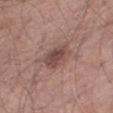Imaged during a routine full-body skin examination; the lesion was not biopsied and no histopathology is available. About 4.5 mm across. Automated image analysis of the tile measured a border-irregularity index near 2.5/10, a color-variation rating of about 3.5/10, and a peripheral color-asymmetry measure near 1. The analysis additionally found an automated nevus-likeness rating near 65 out of 100. A roughly 15 mm field-of-view crop from a total-body skin photograph. Imaged with white-light lighting. From the right thigh. A male subject, roughly 70 years of age.